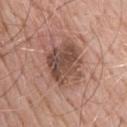<lesion>
  <biopsy_status>not biopsied; imaged during a skin examination</biopsy_status>
  <site>back</site>
  <image>
    <source>total-body photography crop</source>
    <field_of_view_mm>15</field_of_view_mm>
  </image>
  <patient>
    <sex>male</sex>
    <age_approx>65</age_approx>
  </patient>
</lesion>